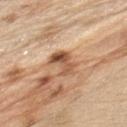Clinical summary: A male patient about 70 years old. This image is a 15 mm lesion crop taken from a total-body photograph. Imaged with white-light lighting. Approximately 3.5 mm at its widest. The lesion is located on the left upper arm.